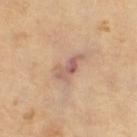Context:
This is a cross-polarized tile. A female patient, approximately 70 years of age. On the right thigh. This image is a 15 mm lesion crop taken from a total-body photograph. Longest diameter approximately 4 mm.